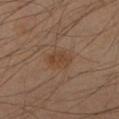Q: Was a biopsy performed?
A: imaged on a skin check; not biopsied
Q: How large is the lesion?
A: about 3 mm
Q: Lesion location?
A: the left forearm
Q: Patient demographics?
A: male, about 55 years old
Q: What did automated image analysis measure?
A: a shape eccentricity near 0.75 and two-axis asymmetry of about 0.2; a lesion–skin lightness drop of about 6 and a normalized lesion–skin contrast near 6.5; a border-irregularity index near 2/10, a within-lesion color-variation index near 2/10, and radial color variation of about 0.5; a classifier nevus-likeness of about 35/100 and a lesion-detection confidence of about 100/100
Q: What is the imaging modality?
A: 15 mm crop, total-body photography